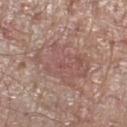Clinical impression:
No biopsy was performed on this lesion — it was imaged during a full skin examination and was not determined to be concerning.
Acquisition and patient details:
The lesion's longest dimension is about 7 mm. A male subject aged around 70. A roughly 15 mm field-of-view crop from a total-body skin photograph. The lesion is located on the right lower leg.